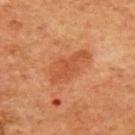• follow-up — no biopsy performed (imaged during a skin exam)
• TBP lesion metrics — a footprint of about 11 mm², an eccentricity of roughly 0.9, and two-axis asymmetry of about 0.35; a lesion color around L≈48 a*≈28 b*≈36 in CIELAB, about 7 CIELAB-L* units darker than the surrounding skin, and a normalized border contrast of about 6; a border-irregularity rating of about 4/10 and a within-lesion color-variation index near 2/10; a classifier nevus-likeness of about 45/100 and a detector confidence of about 100 out of 100 that the crop contains a lesion
• anatomic site — the front of the torso
• lighting — cross-polarized
• subject — male, aged 58 to 62
• image — 15 mm crop, total-body photography